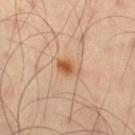workup = no biopsy performed (imaged during a skin exam).